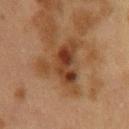Clinical impression: Recorded during total-body skin imaging; not selected for excision or biopsy.